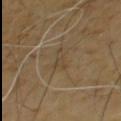Q: Was a biopsy performed?
A: total-body-photography surveillance lesion; no biopsy
Q: Who is the patient?
A: male, aged around 65
Q: Automated lesion metrics?
A: roughly 5 lightness units darker than nearby skin and a lesion-to-skin contrast of about 4.5 (normalized; higher = more distinct)
Q: Illumination type?
A: cross-polarized
Q: Lesion location?
A: the chest
Q: What kind of image is this?
A: total-body-photography crop, ~15 mm field of view